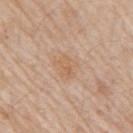Impression:
Captured during whole-body skin photography for melanoma surveillance; the lesion was not biopsied.
Clinical summary:
The subject is a male approximately 80 years of age. A region of skin cropped from a whole-body photographic capture, roughly 15 mm wide. From the right upper arm.